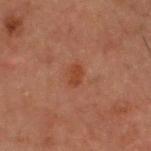image = ~15 mm crop, total-body skin-cancer survey; patient = male, roughly 60 years of age; site = the head or neck.